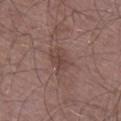follow-up = no biopsy performed (imaged during a skin exam) | lighting = white-light | lesion diameter = ~3.5 mm (longest diameter) | imaging modality = total-body-photography crop, ~15 mm field of view | subject = male, approximately 65 years of age | location = the leg.